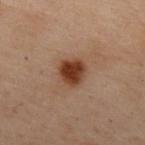workup = no biopsy performed (imaged during a skin exam)